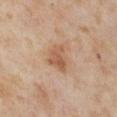This lesion was catalogued during total-body skin photography and was not selected for biopsy. Automated image analysis of the tile measured a lesion area of about 7 mm², an eccentricity of roughly 0.6, and a symmetry-axis asymmetry near 0.35. And it measured about 9 CIELAB-L* units darker than the surrounding skin and a lesion-to-skin contrast of about 6.5 (normalized; higher = more distinct). From the left thigh. A region of skin cropped from a whole-body photographic capture, roughly 15 mm wide. This is a cross-polarized tile. The patient is a female aged 53 to 57. The lesion's longest dimension is about 3.5 mm.